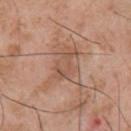notes = no biopsy performed (imaged during a skin exam)
image source = 15 mm crop, total-body photography
lighting = white-light
size = about 4 mm
image-analysis metrics = a lesion color around L≈53 a*≈20 b*≈29 in CIELAB, about 7 CIELAB-L* units darker than the surrounding skin, and a normalized lesion–skin contrast near 5; a nevus-likeness score of about 0/100 and a detector confidence of about 95 out of 100 that the crop contains a lesion
body site = the upper back
subject = male, roughly 55 years of age